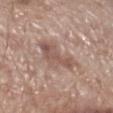Clinical impression: No biopsy was performed on this lesion — it was imaged during a full skin examination and was not determined to be concerning. Context: A male patient, aged approximately 70. The lesion is located on the leg. The recorded lesion diameter is about 5 mm. Cropped from a total-body skin-imaging series; the visible field is about 15 mm. The lesion-visualizer software estimated a lesion color around L≈54 a*≈18 b*≈24 in CIELAB, about 9 CIELAB-L* units darker than the surrounding skin, and a lesion-to-skin contrast of about 6.5 (normalized; higher = more distinct). The analysis additionally found a classifier nevus-likeness of about 0/100.